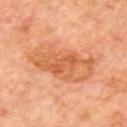Captured during whole-body skin photography for melanoma surveillance; the lesion was not biopsied.
Longest diameter approximately 8 mm.
Imaged with cross-polarized lighting.
Automated tile analysis of the lesion measured a footprint of about 25 mm² and an eccentricity of roughly 0.9. The software also gave an average lesion color of about L≈60 a*≈28 b*≈40 (CIELAB), a lesion–skin lightness drop of about 9, and a normalized border contrast of about 6.5.
This image is a 15 mm lesion crop taken from a total-body photograph.
The subject is a male aged 58 to 62.
The lesion is located on the upper back.